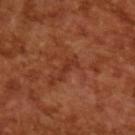Recorded during total-body skin imaging; not selected for excision or biopsy.
A 15 mm crop from a total-body photograph taken for skin-cancer surveillance.
Automated image analysis of the tile measured a mean CIELAB color near L≈33 a*≈27 b*≈31, a lesion–skin lightness drop of about 6, and a lesion-to-skin contrast of about 6 (normalized; higher = more distinct). The analysis additionally found a border-irregularity rating of about 6/10, internal color variation of about 0 on a 0–10 scale, and a peripheral color-asymmetry measure near 0.
Captured under cross-polarized illumination.
A male subject roughly 65 years of age.